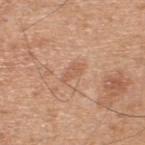automated_metrics:
  eccentricity: 0.9
  shape_asymmetry: 0.3
  border_irregularity_0_10: 3.0
  color_variation_0_10: 1.0
  peripheral_color_asymmetry: 0.0
  nevus_likeness_0_100: 0
lesion_size:
  long_diameter_mm_approx: 3.0
image:
  source: total-body photography crop
  field_of_view_mm: 15
patient:
  sex: male
  age_approx: 45
site: back